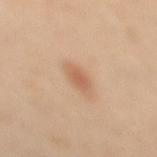Clinical impression: Captured during whole-body skin photography for melanoma surveillance; the lesion was not biopsied. Acquisition and patient details: This is a cross-polarized tile. This image is a 15 mm lesion crop taken from a total-body photograph. Located on the left leg. A female subject about 40 years old. Longest diameter approximately 2.5 mm.